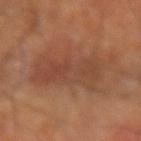Captured during whole-body skin photography for melanoma surveillance; the lesion was not biopsied.
A roughly 15 mm field-of-view crop from a total-body skin photograph.
The total-body-photography lesion software estimated a footprint of about 29 mm² and a symmetry-axis asymmetry near 0.25. It also reported a mean CIELAB color near L≈43 a*≈22 b*≈30, roughly 7 lightness units darker than nearby skin, and a normalized lesion–skin contrast near 5.5. It also reported a color-variation rating of about 4.5/10 and peripheral color asymmetry of about 1.5. And it measured a classifier nevus-likeness of about 10/100.
The tile uses cross-polarized illumination.
On the arm.
The subject is a male roughly 70 years of age.
The recorded lesion diameter is about 8.5 mm.